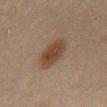Impression:
This lesion was catalogued during total-body skin photography and was not selected for biopsy.
Acquisition and patient details:
A male subject, aged around 45. The lesion is located on the abdomen. Imaged with cross-polarized lighting. A 15 mm crop from a total-body photograph taken for skin-cancer surveillance. Approximately 5 mm at its widest.